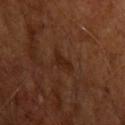Clinical impression:
The lesion was tiled from a total-body skin photograph and was not biopsied.
Context:
On the left upper arm. The patient is a male about 65 years old. Cropped from a whole-body photographic skin survey; the tile spans about 15 mm.